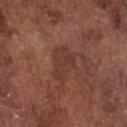| key | value |
|---|---|
| biopsy status | no biopsy performed (imaged during a skin exam) |
| subject | male, in their mid-70s |
| location | the front of the torso |
| image source | total-body-photography crop, ~15 mm field of view |
| lesion diameter | ~4.5 mm (longest diameter) |
| automated metrics | an average lesion color of about L≈37 a*≈22 b*≈25 (CIELAB) and about 6 CIELAB-L* units darker than the surrounding skin; border irregularity of about 3.5 on a 0–10 scale and a within-lesion color-variation index near 2/10 |
| illumination | white-light illumination |